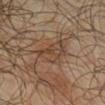This lesion was catalogued during total-body skin photography and was not selected for biopsy. From the right lower leg. The subject is a male approximately 65 years of age. A close-up tile cropped from a whole-body skin photograph, about 15 mm across.